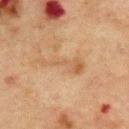Assessment: The lesion was tiled from a total-body skin photograph and was not biopsied. Background: The tile uses cross-polarized illumination. A male patient roughly 70 years of age. The lesion is on the upper back. Cropped from a total-body skin-imaging series; the visible field is about 15 mm. Longest diameter approximately 6.5 mm.